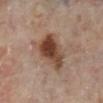Recorded during total-body skin imaging; not selected for excision or biopsy.
The lesion is on the leg.
Automated image analysis of the tile measured an area of roughly 16 mm², a shape eccentricity near 0.65, and a symmetry-axis asymmetry near 0.3. The analysis additionally found an average lesion color of about L≈44 a*≈19 b*≈27 (CIELAB) and roughly 13 lightness units darker than nearby skin. The software also gave an automated nevus-likeness rating near 95 out of 100 and a lesion-detection confidence of about 100/100.
The patient is a female aged around 60.
Cropped from a whole-body photographic skin survey; the tile spans about 15 mm.
The recorded lesion diameter is about 5 mm.
The tile uses cross-polarized illumination.